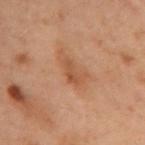Assessment: Captured during whole-body skin photography for melanoma surveillance; the lesion was not biopsied. Image and clinical context: Imaged with cross-polarized lighting. A roughly 15 mm field-of-view crop from a total-body skin photograph. Longest diameter approximately 3 mm. Automated image analysis of the tile measured a lesion color around L≈43 a*≈20 b*≈30 in CIELAB, about 7 CIELAB-L* units darker than the surrounding skin, and a lesion-to-skin contrast of about 6 (normalized; higher = more distinct). A female patient, aged 53–57. Located on the left arm.